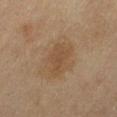notes = no biopsy performed (imaged during a skin exam) | subject = female, aged approximately 60 | size = ~3.5 mm (longest diameter) | illumination = cross-polarized | imaging modality = total-body-photography crop, ~15 mm field of view | body site = the left thigh.